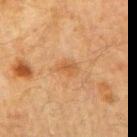A male patient roughly 70 years of age. Automated image analysis of the tile measured an area of roughly 4 mm², an outline eccentricity of about 0.75 (0 = round, 1 = elongated), and a shape-asymmetry score of about 0.35 (0 = symmetric). The software also gave an average lesion color of about L≈48 a*≈20 b*≈35 (CIELAB) and roughly 6 lightness units darker than nearby skin. It also reported a border-irregularity index near 3/10 and a color-variation rating of about 2.5/10. The software also gave a classifier nevus-likeness of about 15/100 and lesion-presence confidence of about 100/100. The tile uses cross-polarized illumination. A 15 mm close-up extracted from a 3D total-body photography capture. The lesion is on the left upper arm. Longest diameter approximately 2.5 mm.